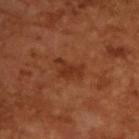Recorded during total-body skin imaging; not selected for excision or biopsy. A male patient, in their mid-60s. A roughly 15 mm field-of-view crop from a total-body skin photograph. The tile uses cross-polarized illumination.